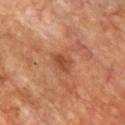Q: Was this lesion biopsied?
A: total-body-photography surveillance lesion; no biopsy
Q: Automated lesion metrics?
A: a lesion area of about 4 mm² and a symmetry-axis asymmetry near 0.25; a lesion-detection confidence of about 100/100
Q: Lesion size?
A: about 2.5 mm
Q: How was this image acquired?
A: ~15 mm crop, total-body skin-cancer survey
Q: Illumination type?
A: cross-polarized
Q: What is the anatomic site?
A: the front of the torso
Q: Patient demographics?
A: male, about 60 years old